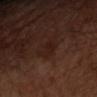Q: Was this lesion biopsied?
A: no biopsy performed (imaged during a skin exam)
Q: How large is the lesion?
A: ≈3 mm
Q: What is the anatomic site?
A: the left forearm
Q: Illumination type?
A: cross-polarized
Q: What kind of image is this?
A: ~15 mm crop, total-body skin-cancer survey
Q: What did automated image analysis measure?
A: a footprint of about 4 mm², an outline eccentricity of about 0.8 (0 = round, 1 = elongated), and a symmetry-axis asymmetry near 0.3; a border-irregularity index near 3/10, a within-lesion color-variation index near 1/10, and peripheral color asymmetry of about 0.5; a classifier nevus-likeness of about 5/100 and lesion-presence confidence of about 100/100
Q: What are the patient's age and sex?
A: male, aged approximately 65